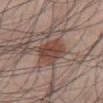Recorded during total-body skin imaging; not selected for excision or biopsy. A male patient roughly 45 years of age. Located on the abdomen. Longest diameter approximately 4 mm. A 15 mm close-up tile from a total-body photography series done for melanoma screening.